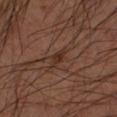Clinical impression:
This lesion was catalogued during total-body skin photography and was not selected for biopsy.
Background:
The lesion is located on the arm. This is a cross-polarized tile. Longest diameter approximately 3 mm. Automated image analysis of the tile measured an average lesion color of about L≈29 a*≈17 b*≈24 (CIELAB). A lesion tile, about 15 mm wide, cut from a 3D total-body photograph. A male patient, in their mid-60s.